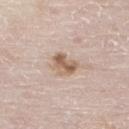Findings:
• follow-up — total-body-photography surveillance lesion; no biopsy
• image source — ~15 mm tile from a whole-body skin photo
• anatomic site — the right lower leg
• illumination — white-light
• patient — male, in their 80s
• size — ≈3.5 mm